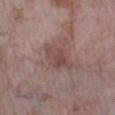<case>
<biopsy_status>not biopsied; imaged during a skin examination</biopsy_status>
<patient>
  <sex>female</sex>
  <age_approx>75</age_approx>
</patient>
<image>
  <source>total-body photography crop</source>
  <field_of_view_mm>15</field_of_view_mm>
</image>
<lighting>white-light</lighting>
<lesion_size>
  <long_diameter_mm_approx>4.5</long_diameter_mm_approx>
</lesion_size>
<automated_metrics>
  <cielab_L>47</cielab_L>
  <cielab_a>18</cielab_a>
  <cielab_b>22</cielab_b>
  <vs_skin_darker_L>8.0</vs_skin_darker_L>
  <vs_skin_contrast_norm>6.0</vs_skin_contrast_norm>
  <lesion_detection_confidence_0_100>100</lesion_detection_confidence_0_100>
</automated_metrics>
<site>left lower leg</site>
</case>